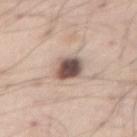{"biopsy_status": "not biopsied; imaged during a skin examination", "image": {"source": "total-body photography crop", "field_of_view_mm": 15}, "patient": {"sex": "male", "age_approx": 55}, "automated_metrics": {"cielab_L": 52, "cielab_a": 16, "cielab_b": 22, "vs_skin_darker_L": 19.0, "vs_skin_contrast_norm": 12.5, "nevus_likeness_0_100": 95, "lesion_detection_confidence_0_100": 100}, "site": "abdomen"}